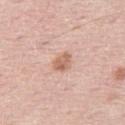Imaged during a routine full-body skin examination; the lesion was not biopsied and no histopathology is available.
A 15 mm crop from a total-body photograph taken for skin-cancer surveillance.
On the right thigh.
A female subject roughly 65 years of age.
This is a white-light tile.